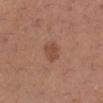Findings:
- follow-up · no biopsy performed (imaged during a skin exam)
- site · the left lower leg
- patient · female, aged 28–32
- image · ~15 mm crop, total-body skin-cancer survey